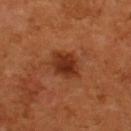biopsy status: no biopsy performed (imaged during a skin exam) | automated lesion analysis: a mean CIELAB color near L≈30 a*≈25 b*≈31 and a lesion–skin lightness drop of about 10; border irregularity of about 2 on a 0–10 scale, a color-variation rating of about 3.5/10, and a peripheral color-asymmetry measure near 1 | imaging modality: total-body-photography crop, ~15 mm field of view | body site: the upper back | patient: male, in their mid-50s | diameter: ≈3.5 mm.